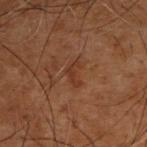biopsy_status: not biopsied; imaged during a skin examination
image:
  source: total-body photography crop
  field_of_view_mm: 15
patient:
  sex: male
  age_approx: 50
site: upper back
lesion_size:
  long_diameter_mm_approx: 3.0